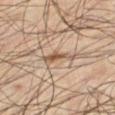• workup · imaged on a skin check; not biopsied